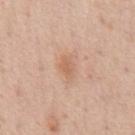| feature | finding |
|---|---|
| follow-up | total-body-photography surveillance lesion; no biopsy |
| tile lighting | white-light illumination |
| body site | the front of the torso |
| acquisition | ~15 mm crop, total-body skin-cancer survey |
| patient | male, about 55 years old |